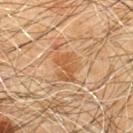Impression: Part of a total-body skin-imaging series; this lesion was reviewed on a skin check and was not flagged for biopsy. Acquisition and patient details: A 15 mm close-up extracted from a 3D total-body photography capture. On the chest. About 3.5 mm across. A male patient, about 60 years old.